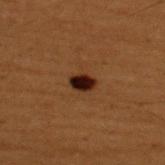The lesion was photographed on a routine skin check and not biopsied; there is no pathology result.
Captured under cross-polarized illumination.
About 2.5 mm across.
An algorithmic analysis of the crop reported an average lesion color of about L≈17 a*≈17 b*≈20 (CIELAB). The analysis additionally found peripheral color asymmetry of about 1. It also reported a nevus-likeness score of about 100/100 and a detector confidence of about 100 out of 100 that the crop contains a lesion.
This image is a 15 mm lesion crop taken from a total-body photograph.
Located on the upper back.
A male subject aged 48 to 52.